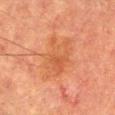Case summary:
- biopsy status · no biopsy performed (imaged during a skin exam)
- size · about 6.5 mm
- patient · female, about 55 years old
- anatomic site · the right forearm
- image source · ~15 mm crop, total-body skin-cancer survey
- tile lighting · cross-polarized